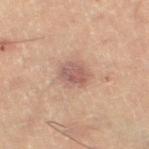Q: Was this lesion biopsied?
A: imaged on a skin check; not biopsied
Q: What are the patient's age and sex?
A: male, aged 58 to 62
Q: What is the imaging modality?
A: 15 mm crop, total-body photography
Q: What lighting was used for the tile?
A: cross-polarized
Q: What is the lesion's diameter?
A: ~3.5 mm (longest diameter)